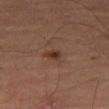The lesion was tiled from a total-body skin photograph and was not biopsied. Cropped from a whole-body photographic skin survey; the tile spans about 15 mm. A male subject, approximately 65 years of age. The lesion is on the left thigh. This is a cross-polarized tile. The lesion's longest dimension is about 2 mm.